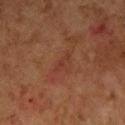biopsy_status: not biopsied; imaged during a skin examination
image:
  source: total-body photography crop
  field_of_view_mm: 15
automated_metrics:
  nevus_likeness_0_100: 0
patient:
  sex: female
  age_approx: 60
site: left forearm